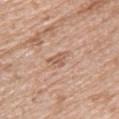Assessment: This lesion was catalogued during total-body skin photography and was not selected for biopsy. Acquisition and patient details: On the back. A female patient roughly 70 years of age. A 15 mm close-up extracted from a 3D total-body photography capture.